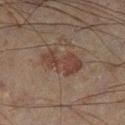The lesion was photographed on a routine skin check and not biopsied; there is no pathology result. Located on the leg. The patient is a male aged 58 to 62. Automated tile analysis of the lesion measured an average lesion color of about L≈29 a*≈14 b*≈19 (CIELAB), about 7 CIELAB-L* units darker than the surrounding skin, and a lesion-to-skin contrast of about 7 (normalized; higher = more distinct). The software also gave a detector confidence of about 100 out of 100 that the crop contains a lesion. A 15 mm close-up tile from a total-body photography series done for melanoma screening.